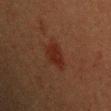Context:
Cropped from a total-body skin-imaging series; the visible field is about 15 mm. A male subject in their 40s. The tile uses cross-polarized illumination. Located on the chest. Approximately 3 mm at its widest.